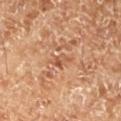Findings:
- notes — imaged on a skin check; not biopsied
- diameter — ≈3 mm
- TBP lesion metrics — an eccentricity of roughly 0.8 and two-axis asymmetry of about 0.35; a lesion color around L≈53 a*≈24 b*≈34 in CIELAB and a normalized border contrast of about 5.5; a classifier nevus-likeness of about 0/100
- imaging modality — ~15 mm crop, total-body skin-cancer survey
- site — the left lower leg
- subject — male, about 70 years old
- tile lighting — cross-polarized